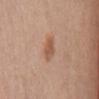Imaged during a routine full-body skin examination; the lesion was not biopsied and no histopathology is available.
A female subject about 65 years old.
Imaged with white-light lighting.
The recorded lesion diameter is about 3 mm.
A region of skin cropped from a whole-body photographic capture, roughly 15 mm wide.
Automated tile analysis of the lesion measured a mean CIELAB color near L≈55 a*≈22 b*≈31 and a lesion–skin lightness drop of about 9.
From the mid back.